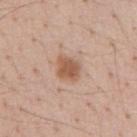Assessment: Captured during whole-body skin photography for melanoma surveillance; the lesion was not biopsied. Background: The lesion is on the chest. A roughly 15 mm field-of-view crop from a total-body skin photograph. Measured at roughly 2.5 mm in maximum diameter. A male patient aged around 55. The tile uses white-light illumination.